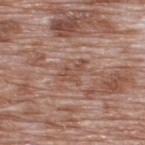The lesion was tiled from a total-body skin photograph and was not biopsied. A lesion tile, about 15 mm wide, cut from a 3D total-body photograph. From the upper back. A male subject approximately 70 years of age.